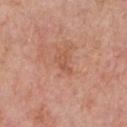Notes:
* workup · imaged on a skin check; not biopsied
* imaging modality · ~15 mm tile from a whole-body skin photo
* location · the chest
* subject · male, about 80 years old